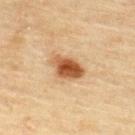The recorded lesion diameter is about 4.5 mm.
Located on the upper back.
The tile uses cross-polarized illumination.
An algorithmic analysis of the crop reported a shape eccentricity near 0.8 and a symmetry-axis asymmetry near 0.25. The analysis additionally found an average lesion color of about L≈47 a*≈20 b*≈34 (CIELAB), a lesion–skin lightness drop of about 15, and a lesion-to-skin contrast of about 11 (normalized; higher = more distinct). It also reported a border-irregularity index near 2.5/10, a within-lesion color-variation index near 6.5/10, and a peripheral color-asymmetry measure near 2.
A region of skin cropped from a whole-body photographic capture, roughly 15 mm wide.
A male subject, aged approximately 45.